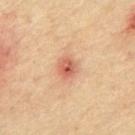Captured during whole-body skin photography for melanoma surveillance; the lesion was not biopsied.
Located on the front of the torso.
The patient is a male aged approximately 65.
About 3 mm across.
The total-body-photography lesion software estimated a lesion area of about 5 mm² and two-axis asymmetry of about 0.2. And it measured a mean CIELAB color near L≈54 a*≈24 b*≈30, a lesion–skin lightness drop of about 11, and a lesion-to-skin contrast of about 7.5 (normalized; higher = more distinct).
Cropped from a whole-body photographic skin survey; the tile spans about 15 mm.
The tile uses cross-polarized illumination.